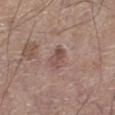Imaged during a routine full-body skin examination; the lesion was not biopsied and no histopathology is available. This image is a 15 mm lesion crop taken from a total-body photograph. The tile uses white-light illumination. From the left lower leg. The patient is a male roughly 65 years of age.